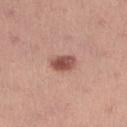This lesion was catalogued during total-body skin photography and was not selected for biopsy.
On the right lower leg.
A female subject about 25 years old.
Cropped from a total-body skin-imaging series; the visible field is about 15 mm.